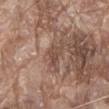<tbp_lesion>
  <biopsy_status>not biopsied; imaged during a skin examination</biopsy_status>
  <patient>
    <sex>male</sex>
    <age_approx>80</age_approx>
  </patient>
  <image>
    <source>total-body photography crop</source>
    <field_of_view_mm>15</field_of_view_mm>
  </image>
  <lesion_size>
    <long_diameter_mm_approx>2.5</long_diameter_mm_approx>
  </lesion_size>
  <site>abdomen</site>
  <automated_metrics>
    <area_mm2_approx>3.0</area_mm2_approx>
    <eccentricity>0.8</eccentricity>
    <shape_asymmetry>0.4</shape_asymmetry>
    <cielab_L>47</cielab_L>
    <cielab_a>19</cielab_a>
    <cielab_b>26</cielab_b>
    <vs_skin_darker_L>8.0</vs_skin_darker_L>
    <vs_skin_contrast_norm>6.0</vs_skin_contrast_norm>
    <border_irregularity_0_10>4.0</border_irregularity_0_10>
    <peripheral_color_asymmetry>0.5</peripheral_color_asymmetry>
    <nevus_likeness_0_100>0</nevus_likeness_0_100>
    <lesion_detection_confidence_0_100>95</lesion_detection_confidence_0_100>
  </automated_metrics>
</tbp_lesion>